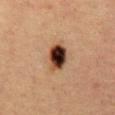Recorded during total-body skin imaging; not selected for excision or biopsy.
The total-body-photography lesion software estimated a lesion area of about 8 mm², a shape eccentricity near 0.7, and a symmetry-axis asymmetry near 0.15. The analysis additionally found a mean CIELAB color near L≈30 a*≈18 b*≈24, roughly 21 lightness units darker than nearby skin, and a normalized lesion–skin contrast near 17.5.
A female patient approximately 40 years of age.
Captured under cross-polarized illumination.
Measured at roughly 3.5 mm in maximum diameter.
A 15 mm close-up extracted from a 3D total-body photography capture.
From the chest.